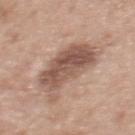Clinical impression: The lesion was photographed on a routine skin check and not biopsied; there is no pathology result. Clinical summary: This is a white-light tile. Automated image analysis of the tile measured roughly 13 lightness units darker than nearby skin and a normalized lesion–skin contrast near 8.5. It also reported a border-irregularity index near 4.5/10, a within-lesion color-variation index near 5/10, and a peripheral color-asymmetry measure near 2. A 15 mm close-up tile from a total-body photography series done for melanoma screening. Located on the back. Approximately 6 mm at its widest. A female subject approximately 50 years of age.